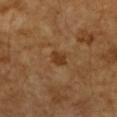notes: imaged on a skin check; not biopsied
image source: total-body-photography crop, ~15 mm field of view
anatomic site: the right forearm
patient: female, in their 70s
lighting: cross-polarized
size: ≈2 mm